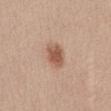<record>
  <biopsy_status>not biopsied; imaged during a skin examination</biopsy_status>
</record>